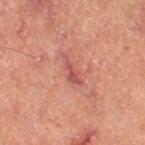biopsy status: no biopsy performed (imaged during a skin exam)
imaging modality: ~15 mm crop, total-body skin-cancer survey
location: the leg
subject: female, approximately 60 years of age